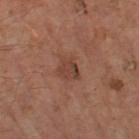A male patient, aged 63 to 67. This image is a 15 mm lesion crop taken from a total-body photograph. The lesion is on the left thigh.